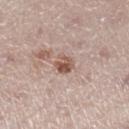Automated image analysis of the tile measured roughly 12 lightness units darker than nearby skin and a lesion-to-skin contrast of about 8.5 (normalized; higher = more distinct). It also reported border irregularity of about 3 on a 0–10 scale and radial color variation of about 2. And it measured a nevus-likeness score of about 30/100 and a detector confidence of about 100 out of 100 that the crop contains a lesion.
Captured under white-light illumination.
On the right lower leg.
A 15 mm crop from a total-body photograph taken for skin-cancer surveillance.
The subject is a female roughly 50 years of age.